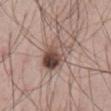Q: Is there a histopathology result?
A: imaged on a skin check; not biopsied